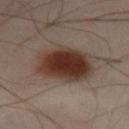biopsy status = no biopsy performed (imaged during a skin exam); lesion size = ≈6.5 mm; acquisition = 15 mm crop, total-body photography; patient = male, in their mid- to late 40s; illumination = cross-polarized; anatomic site = the leg.